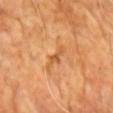Assessment: Captured during whole-body skin photography for melanoma surveillance; the lesion was not biopsied. Background: The lesion is on the chest. A 15 mm close-up extracted from a 3D total-body photography capture. The patient is a male about 70 years old.